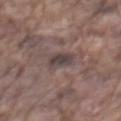| key | value |
|---|---|
| notes | no biopsy performed (imaged during a skin exam) |
| tile lighting | white-light illumination |
| acquisition | ~15 mm crop, total-body skin-cancer survey |
| subject | male, in their mid-70s |
| location | the mid back |